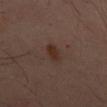Impression: The lesion was photographed on a routine skin check and not biopsied; there is no pathology result. Background: Automated tile analysis of the lesion measured border irregularity of about 2.5 on a 0–10 scale and a color-variation rating of about 3/10. It also reported a nevus-likeness score of about 70/100 and lesion-presence confidence of about 100/100. The patient is a male approximately 50 years of age. A 15 mm crop from a total-body photograph taken for skin-cancer surveillance. Located on the mid back.